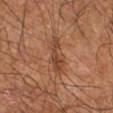Cropped from a whole-body photographic skin survey; the tile spans about 15 mm. This is a cross-polarized tile. A male subject aged around 65. The recorded lesion diameter is about 5 mm. The lesion is on the right upper arm.